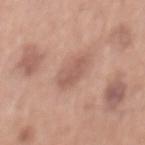No biopsy was performed on this lesion — it was imaged during a full skin examination and was not determined to be concerning.
This is a white-light tile.
Automated tile analysis of the lesion measured an area of roughly 7 mm² and an eccentricity of roughly 0.85.
The subject is a male aged around 70.
From the mid back.
A region of skin cropped from a whole-body photographic capture, roughly 15 mm wide.